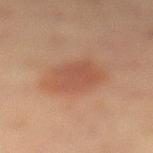Impression: Part of a total-body skin-imaging series; this lesion was reviewed on a skin check and was not flagged for biopsy. Context: From the left lower leg. The recorded lesion diameter is about 4.5 mm. A female subject, aged 18 to 22. Automated image analysis of the tile measured a classifier nevus-likeness of about 100/100 and a detector confidence of about 100 out of 100 that the crop contains a lesion. Cropped from a whole-body photographic skin survey; the tile spans about 15 mm.